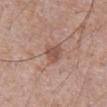| key | value |
|---|---|
| follow-up | total-body-photography surveillance lesion; no biopsy |
| subject | male, roughly 70 years of age |
| body site | the chest |
| diameter | ≈2.5 mm |
| imaging modality | ~15 mm tile from a whole-body skin photo |